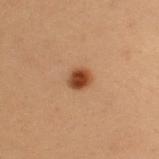Captured during whole-body skin photography for melanoma surveillance; the lesion was not biopsied.
A female patient, approximately 40 years of age.
Imaged with cross-polarized lighting.
Longest diameter approximately 2.5 mm.
On the right upper arm.
A 15 mm close-up extracted from a 3D total-body photography capture.